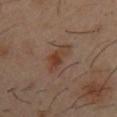Longest diameter approximately 4.5 mm. An algorithmic analysis of the crop reported an average lesion color of about L≈38 a*≈18 b*≈26 (CIELAB), about 7 CIELAB-L* units darker than the surrounding skin, and a lesion-to-skin contrast of about 7 (normalized; higher = more distinct). Captured under cross-polarized illumination. A male subject approximately 40 years of age. The lesion is on the chest. A lesion tile, about 15 mm wide, cut from a 3D total-body photograph.